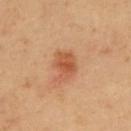Recorded during total-body skin imaging; not selected for excision or biopsy. A 15 mm crop from a total-body photograph taken for skin-cancer surveillance. The lesion is located on the upper back. A male subject, approximately 55 years of age. Captured under cross-polarized illumination. An algorithmic analysis of the crop reported a lesion area of about 7.5 mm², an eccentricity of roughly 0.6, and two-axis asymmetry of about 0.3. It also reported border irregularity of about 3 on a 0–10 scale and a peripheral color-asymmetry measure near 1. The software also gave an automated nevus-likeness rating near 90 out of 100 and a lesion-detection confidence of about 100/100.